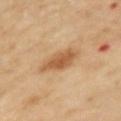Imaged during a routine full-body skin examination; the lesion was not biopsied and no histopathology is available.